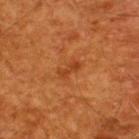biopsy status — catalogued during a skin exam; not biopsied | automated lesion analysis — a mean CIELAB color near L≈39 a*≈29 b*≈40, a lesion–skin lightness drop of about 7, and a lesion-to-skin contrast of about 6 (normalized; higher = more distinct); a classifier nevus-likeness of about 0/100 | patient — male, approximately 60 years of age | illumination — cross-polarized illumination | imaging modality — total-body-photography crop, ~15 mm field of view | anatomic site — the upper back | lesion diameter — ≈2.5 mm.